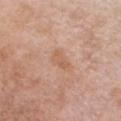{
  "biopsy_status": "not biopsied; imaged during a skin examination",
  "patient": {
    "sex": "female",
    "age_approx": 65
  },
  "image": {
    "source": "total-body photography crop",
    "field_of_view_mm": 15
  },
  "site": "chest"
}